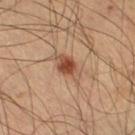notes = no biopsy performed (imaged during a skin exam) | subject = male, aged 38 to 42 | site = the right thigh | image source = ~15 mm crop, total-body skin-cancer survey.